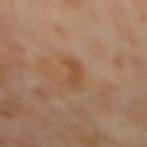Q: Was a biopsy performed?
A: total-body-photography surveillance lesion; no biopsy
Q: Who is the patient?
A: female, in their mid-50s
Q: What is the imaging modality?
A: ~15 mm tile from a whole-body skin photo
Q: Lesion location?
A: the mid back